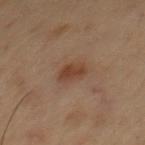biopsy status = imaged on a skin check; not biopsied
automated metrics = a shape eccentricity near 0.6 and two-axis asymmetry of about 0.15
acquisition = 15 mm crop, total-body photography
lesion size = about 3 mm
location = the chest
patient = male, aged 53 to 57
illumination = cross-polarized illumination